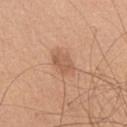Q: Was a biopsy performed?
A: imaged on a skin check; not biopsied
Q: Lesion size?
A: ≈3 mm
Q: Patient demographics?
A: male, aged around 60
Q: What kind of image is this?
A: total-body-photography crop, ~15 mm field of view
Q: Lesion location?
A: the chest
Q: How was the tile lit?
A: white-light